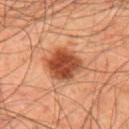Imaged during a routine full-body skin examination; the lesion was not biopsied and no histopathology is available. A roughly 15 mm field-of-view crop from a total-body skin photograph. Captured under cross-polarized illumination. Automated image analysis of the tile measured a lesion area of about 13 mm², a shape eccentricity near 0.35, and a shape-asymmetry score of about 0.15 (0 = symmetric). It also reported an average lesion color of about L≈46 a*≈28 b*≈35 (CIELAB) and a normalized lesion–skin contrast near 11. And it measured a border-irregularity rating of about 1.5/10, a within-lesion color-variation index near 5.5/10, and a peripheral color-asymmetry measure near 2. And it measured a classifier nevus-likeness of about 100/100 and a lesion-detection confidence of about 100/100. From the mid back. A male patient in their mid-40s.